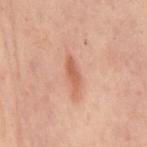Captured during whole-body skin photography for melanoma surveillance; the lesion was not biopsied.
The patient is a female aged 53–57.
Cropped from a whole-body photographic skin survey; the tile spans about 15 mm.
Approximately 4 mm at its widest.
The tile uses cross-polarized illumination.
The lesion is located on the mid back.
Automated image analysis of the tile measured about 9 CIELAB-L* units darker than the surrounding skin and a lesion-to-skin contrast of about 7 (normalized; higher = more distinct). It also reported lesion-presence confidence of about 95/100.